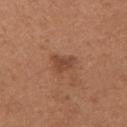location — the right upper arm | automated metrics — an average lesion color of about L≈45 a*≈23 b*≈31 (CIELAB), about 9 CIELAB-L* units darker than the surrounding skin, and a normalized lesion–skin contrast near 6.5; a nevus-likeness score of about 35/100 and lesion-presence confidence of about 100/100 | image source — 15 mm crop, total-body photography | size — about 2.5 mm | illumination — white-light illumination | patient — female, aged 28 to 32.